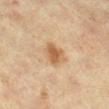Impression:
This lesion was catalogued during total-body skin photography and was not selected for biopsy.
Context:
Captured under cross-polarized illumination. Measured at roughly 3 mm in maximum diameter. A female patient aged around 40. The lesion is on the leg. This image is a 15 mm lesion crop taken from a total-body photograph. An algorithmic analysis of the crop reported a lesion area of about 6.5 mm², a shape eccentricity near 0.45, and a symmetry-axis asymmetry near 0.2. The analysis additionally found an automated nevus-likeness rating near 95 out of 100 and a lesion-detection confidence of about 100/100.